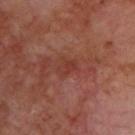This lesion was catalogued during total-body skin photography and was not selected for biopsy.
The lesion is located on the upper back.
This is a cross-polarized tile.
An algorithmic analysis of the crop reported an outline eccentricity of about 0.7 (0 = round, 1 = elongated) and a symmetry-axis asymmetry near 0.3. And it measured about 6 CIELAB-L* units darker than the surrounding skin and a lesion-to-skin contrast of about 5.5 (normalized; higher = more distinct). And it measured a peripheral color-asymmetry measure near 0.5. The analysis additionally found an automated nevus-likeness rating near 0 out of 100 and a lesion-detection confidence of about 100/100.
Cropped from a total-body skin-imaging series; the visible field is about 15 mm.
A male patient aged 68–72.